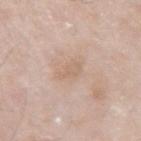automated metrics: roughly 6 lightness units darker than nearby skin and a lesion-to-skin contrast of about 4.5 (normalized; higher = more distinct) | subject: male, about 75 years old | image source: 15 mm crop, total-body photography | anatomic site: the right upper arm | size: about 3 mm | lighting: white-light.